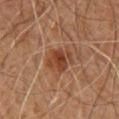biopsy status = no biopsy performed (imaged during a skin exam); diameter = about 3.5 mm; site = the front of the torso; patient = male, in their mid-60s; imaging modality = ~15 mm crop, total-body skin-cancer survey; lighting = cross-polarized illumination.